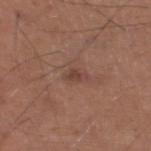Acquisition and patient details: About 2.5 mm across. An algorithmic analysis of the crop reported a footprint of about 3 mm², a shape eccentricity near 0.85, and a symmetry-axis asymmetry near 0.3. It also reported a peripheral color-asymmetry measure near 0.5. The analysis additionally found a nevus-likeness score of about 5/100 and a detector confidence of about 100 out of 100 that the crop contains a lesion. Imaged with white-light lighting. A male patient, in their mid- to late 60s. Cropped from a whole-body photographic skin survey; the tile spans about 15 mm. On the right lower leg.